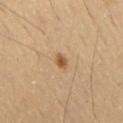follow-up = catalogued during a skin exam; not biopsied | image = ~15 mm tile from a whole-body skin photo | tile lighting = cross-polarized illumination | image-analysis metrics = an average lesion color of about L≈52 a*≈19 b*≈35 (CIELAB), roughly 11 lightness units darker than nearby skin, and a normalized lesion–skin contrast near 8.5; a classifier nevus-likeness of about 90/100 and a detector confidence of about 100 out of 100 that the crop contains a lesion | lesion size = ≈2 mm | patient = male, about 55 years old | anatomic site = the back.